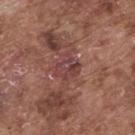Findings:
- workup — total-body-photography surveillance lesion; no biopsy
- body site — the back
- automated metrics — an outline eccentricity of about 0.55 (0 = round, 1 = elongated) and a shape-asymmetry score of about 0.3 (0 = symmetric); a lesion–skin lightness drop of about 7 and a lesion-to-skin contrast of about 7.5 (normalized; higher = more distinct); border irregularity of about 3 on a 0–10 scale and a peripheral color-asymmetry measure near 2; a nevus-likeness score of about 0/100 and lesion-presence confidence of about 65/100
- patient — male, aged approximately 75
- image — ~15 mm crop, total-body skin-cancer survey
- diameter — about 3 mm
- tile lighting — white-light illumination